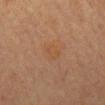Impression:
This lesion was catalogued during total-body skin photography and was not selected for biopsy.
Image and clinical context:
A close-up tile cropped from a whole-body skin photograph, about 15 mm across. A female subject, aged 58–62. From the mid back.